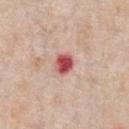The lesion was photographed on a routine skin check and not biopsied; there is no pathology result.
A close-up tile cropped from a whole-body skin photograph, about 15 mm across.
The patient is a male in their 60s.
The lesion is located on the front of the torso.